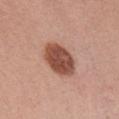Recorded during total-body skin imaging; not selected for excision or biopsy. This is a white-light tile. Automated image analysis of the tile measured a footprint of about 13 mm² and a shape eccentricity near 0.75. It also reported an average lesion color of about L≈49 a*≈24 b*≈29 (CIELAB), roughly 16 lightness units darker than nearby skin, and a normalized border contrast of about 11. The software also gave a within-lesion color-variation index near 4/10. The software also gave a nevus-likeness score of about 90/100 and a lesion-detection confidence of about 100/100. The lesion's longest dimension is about 5 mm. A female subject, roughly 25 years of age. A close-up tile cropped from a whole-body skin photograph, about 15 mm across. On the front of the torso.